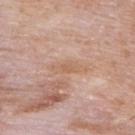Recorded during total-body skin imaging; not selected for excision or biopsy.
About 4 mm across.
Imaged with white-light lighting.
Located on the upper back.
The lesion-visualizer software estimated internal color variation of about 1.5 on a 0–10 scale and a peripheral color-asymmetry measure near 0.5. The analysis additionally found an automated nevus-likeness rating near 0 out of 100.
A roughly 15 mm field-of-view crop from a total-body skin photograph.
A male patient about 60 years old.